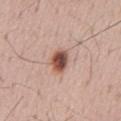Part of a total-body skin-imaging series; this lesion was reviewed on a skin check and was not flagged for biopsy. A male patient, approximately 65 years of age. This is a white-light tile. Cropped from a whole-body photographic skin survey; the tile spans about 15 mm. The total-body-photography lesion software estimated an eccentricity of roughly 0.7 and a symmetry-axis asymmetry near 0.15. The recorded lesion diameter is about 3 mm. On the mid back.